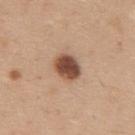Case summary:
- workup · total-body-photography surveillance lesion; no biopsy
- acquisition · total-body-photography crop, ~15 mm field of view
- body site · the mid back
- subject · male, approximately 35 years of age